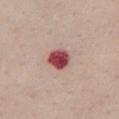Impression:
Recorded during total-body skin imaging; not selected for excision or biopsy.
Background:
An algorithmic analysis of the crop reported a shape eccentricity near 0.4 and a shape-asymmetry score of about 0.15 (0 = symmetric). Imaged with white-light lighting. Longest diameter approximately 3 mm. From the chest. A close-up tile cropped from a whole-body skin photograph, about 15 mm across. A female subject, in their mid- to late 50s.